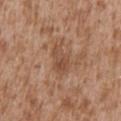{
  "image": {
    "source": "total-body photography crop",
    "field_of_view_mm": 15
  },
  "lighting": "white-light",
  "patient": {
    "sex": "male",
    "age_approx": 45
  },
  "lesion_size": {
    "long_diameter_mm_approx": 3.5
  },
  "site": "abdomen"
}